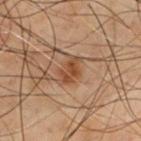| feature | finding |
|---|---|
| biopsy status | no biopsy performed (imaged during a skin exam) |
| diameter | about 3 mm |
| patient | male, aged 68 to 72 |
| automated metrics | an area of roughly 5.5 mm², a shape eccentricity near 0.65, and two-axis asymmetry of about 0.25; a border-irregularity rating of about 3/10, a color-variation rating of about 2.5/10, and a peripheral color-asymmetry measure near 1; a nevus-likeness score of about 40/100 and a detector confidence of about 100 out of 100 that the crop contains a lesion |
| site | the chest |
| image source | ~15 mm crop, total-body skin-cancer survey |